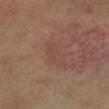{"biopsy_status": "not biopsied; imaged during a skin examination", "image": {"source": "total-body photography crop", "field_of_view_mm": 15}, "patient": {"sex": "female"}, "automated_metrics": {"area_mm2_approx": 6.0, "eccentricity": 0.9, "shape_asymmetry": 0.25, "cielab_L": 46, "cielab_a": 22, "cielab_b": 24, "border_irregularity_0_10": 3.0, "color_variation_0_10": 2.5, "peripheral_color_asymmetry": 1.0, "nevus_likeness_0_100": 0, "lesion_detection_confidence_0_100": 100}}